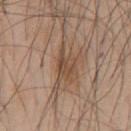This lesion was catalogued during total-body skin photography and was not selected for biopsy.
A 15 mm close-up extracted from a 3D total-body photography capture.
Automated image analysis of the tile measured a shape eccentricity near 0.85. The software also gave a lesion color around L≈47 a*≈17 b*≈29 in CIELAB, a lesion–skin lightness drop of about 9, and a normalized lesion–skin contrast near 7.
The patient is a male in their mid-40s.
From the chest.
Imaged with white-light lighting.